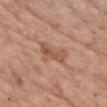Q: Is there a histopathology result?
A: no biopsy performed (imaged during a skin exam)
Q: What did automated image analysis measure?
A: an area of roughly 7 mm² and a shape-asymmetry score of about 0.3 (0 = symmetric); lesion-presence confidence of about 100/100
Q: What is the lesion's diameter?
A: ~4 mm (longest diameter)
Q: What is the anatomic site?
A: the chest
Q: What is the imaging modality?
A: 15 mm crop, total-body photography
Q: What are the patient's age and sex?
A: male, aged 58 to 62